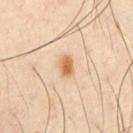<tbp_lesion>
<biopsy_status>not biopsied; imaged during a skin examination</biopsy_status>
<site>front of the torso</site>
<automated_metrics>
  <area_mm2_approx>3.5</area_mm2_approx>
  <shape_asymmetry>0.2</shape_asymmetry>
  <lesion_detection_confidence_0_100>100</lesion_detection_confidence_0_100>
</automated_metrics>
<patient>
  <sex>male</sex>
  <age_approx>45</age_approx>
</patient>
<image>
  <source>total-body photography crop</source>
  <field_of_view_mm>15</field_of_view_mm>
</image>
<lighting>cross-polarized</lighting>
<lesion_size>
  <long_diameter_mm_approx>2.5</long_diameter_mm_approx>
</lesion_size>
</tbp_lesion>